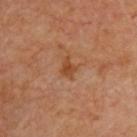Recorded during total-body skin imaging; not selected for excision or biopsy.
A 15 mm close-up extracted from a 3D total-body photography capture.
Automated image analysis of the tile measured an average lesion color of about L≈46 a*≈24 b*≈36 (CIELAB), a lesion–skin lightness drop of about 8, and a lesion-to-skin contrast of about 7 (normalized; higher = more distinct). The analysis additionally found a detector confidence of about 100 out of 100 that the crop contains a lesion.
Imaged with cross-polarized lighting.
From the upper back.
Longest diameter approximately 2.5 mm.
The patient is aged around 65.